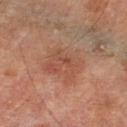biopsy status = imaged on a skin check; not biopsied.